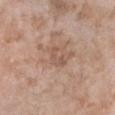<tbp_lesion>
  <biopsy_status>not biopsied; imaged during a skin examination</biopsy_status>
  <lesion_size>
    <long_diameter_mm_approx>3.0</long_diameter_mm_approx>
  </lesion_size>
  <image>
    <source>total-body photography crop</source>
    <field_of_view_mm>15</field_of_view_mm>
  </image>
  <patient>
    <sex>female</sex>
    <age_approx>75</age_approx>
  </patient>
  <lighting>white-light</lighting>
  <automated_metrics>
    <cielab_L>54</cielab_L>
    <cielab_a>18</cielab_a>
    <cielab_b>28</cielab_b>
    <vs_skin_darker_L>7.0</vs_skin_darker_L>
    <vs_skin_contrast_norm>5.0</vs_skin_contrast_norm>
    <nevus_likeness_0_100>0</nevus_likeness_0_100>
    <lesion_detection_confidence_0_100>100</lesion_detection_confidence_0_100>
  </automated_metrics>
  <site>front of the torso</site>
</tbp_lesion>